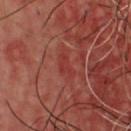Clinical impression: Recorded during total-body skin imaging; not selected for excision or biopsy. Clinical summary: The lesion is on the chest. About 3 mm across. The tile uses cross-polarized illumination. A male subject approximately 50 years of age. Automated image analysis of the tile measured a lesion–skin lightness drop of about 5. And it measured internal color variation of about 0.5 on a 0–10 scale and radial color variation of about 0. The software also gave a classifier nevus-likeness of about 0/100. This image is a 15 mm lesion crop taken from a total-body photograph.